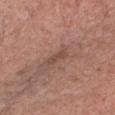Impression: This lesion was catalogued during total-body skin photography and was not selected for biopsy. Clinical summary: A 15 mm close-up extracted from a 3D total-body photography capture. The lesion is located on the chest. The lesion's longest dimension is about 2.5 mm. The patient is a female about 60 years old. Automated tile analysis of the lesion measured a lesion area of about 2.5 mm², an outline eccentricity of about 0.9 (0 = round, 1 = elongated), and two-axis asymmetry of about 0.4. And it measured an automated nevus-likeness rating near 0 out of 100 and a lesion-detection confidence of about 55/100.